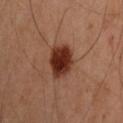notes — imaged on a skin check; not biopsied | site — the left upper arm | image source — ~15 mm crop, total-body skin-cancer survey | patient — male, aged approximately 45.